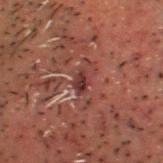Q: Was this lesion biopsied?
A: catalogued during a skin exam; not biopsied
Q: What are the patient's age and sex?
A: male, about 50 years old
Q: What did automated image analysis measure?
A: a lesion color around L≈26 a*≈21 b*≈18 in CIELAB; an automated nevus-likeness rating near 0 out of 100 and lesion-presence confidence of about 55/100
Q: Illumination type?
A: cross-polarized illumination
Q: Where on the body is the lesion?
A: the head or neck
Q: What is the imaging modality?
A: 15 mm crop, total-body photography
Q: How large is the lesion?
A: ~2.5 mm (longest diameter)